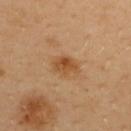follow-up: catalogued during a skin exam; not biopsied
subject: male, aged around 40
lesion size: ~3.5 mm (longest diameter)
anatomic site: the upper back
illumination: cross-polarized illumination
automated metrics: an automated nevus-likeness rating near 85 out of 100 and a detector confidence of about 100 out of 100 that the crop contains a lesion
image: ~15 mm tile from a whole-body skin photo